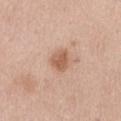biopsy status: total-body-photography surveillance lesion; no biopsy
image: 15 mm crop, total-body photography
anatomic site: the abdomen
image-analysis metrics: an average lesion color of about L≈59 a*≈22 b*≈31 (CIELAB), roughly 11 lightness units darker than nearby skin, and a lesion-to-skin contrast of about 7.5 (normalized; higher = more distinct); a border-irregularity rating of about 2.5/10, a within-lesion color-variation index near 2/10, and a peripheral color-asymmetry measure near 0.5
lighting: white-light
patient: female, aged 58–62
lesion diameter: ~3 mm (longest diameter)